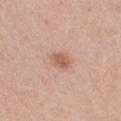Part of a total-body skin-imaging series; this lesion was reviewed on a skin check and was not flagged for biopsy. An algorithmic analysis of the crop reported a lesion area of about 4.5 mm², a shape eccentricity near 0.6, and a symmetry-axis asymmetry near 0.2. The software also gave border irregularity of about 1.5 on a 0–10 scale, a within-lesion color-variation index near 1.5/10, and radial color variation of about 0.5. The analysis additionally found a classifier nevus-likeness of about 80/100 and a detector confidence of about 100 out of 100 that the crop contains a lesion. About 2.5 mm across. Located on the right thigh. A female patient approximately 45 years of age. A close-up tile cropped from a whole-body skin photograph, about 15 mm across.